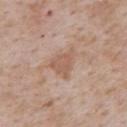Recorded during total-body skin imaging; not selected for excision or biopsy. From the upper back. Measured at roughly 3.5 mm in maximum diameter. A male subject roughly 75 years of age. The total-body-photography lesion software estimated a mean CIELAB color near L≈57 a*≈19 b*≈28 and roughly 8 lightness units darker than nearby skin. And it measured a border-irregularity index near 3.5/10, a color-variation rating of about 2/10, and radial color variation of about 1. A close-up tile cropped from a whole-body skin photograph, about 15 mm across. The tile uses white-light illumination.